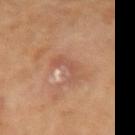Findings:
• workup · catalogued during a skin exam; not biopsied
• subject · female, about 65 years old
• lesion size · about 4.5 mm
• image-analysis metrics · a lesion area of about 5.5 mm² and an eccentricity of roughly 0.95
• imaging modality · ~15 mm tile from a whole-body skin photo
• location · the arm
• lighting · cross-polarized illumination